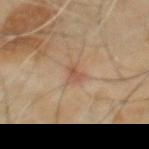This lesion was catalogued during total-body skin photography and was not selected for biopsy. Located on the back. The subject is a male about 60 years old. A 15 mm close-up tile from a total-body photography series done for melanoma screening.